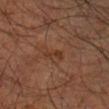Imaged during a routine full-body skin examination; the lesion was not biopsied and no histopathology is available.
A lesion tile, about 15 mm wide, cut from a 3D total-body photograph.
The lesion is located on the left arm.
The recorded lesion diameter is about 3 mm.
The subject is a male in their mid-60s.
This is a cross-polarized tile.